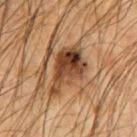<record>
<automated_metrics>
  <area_mm2_approx>15.0</area_mm2_approx>
  <eccentricity>0.75</eccentricity>
  <shape_asymmetry>0.35</shape_asymmetry>
  <vs_skin_darker_L>13.0</vs_skin_darker_L>
  <border_irregularity_0_10>4.0</border_irregularity_0_10>
  <color_variation_0_10>8.5</color_variation_0_10>
  <peripheral_color_asymmetry>3.0</peripheral_color_asymmetry>
  <nevus_likeness_0_100>15</nevus_likeness_0_100>
  <lesion_detection_confidence_0_100>95</lesion_detection_confidence_0_100>
</automated_metrics>
<patient>
  <sex>male</sex>
  <age_approx>65</age_approx>
</patient>
<lighting>cross-polarized</lighting>
<site>right upper arm</site>
<image>
  <source>total-body photography crop</source>
  <field_of_view_mm>15</field_of_view_mm>
</image>
</record>